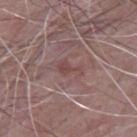Part of a total-body skin-imaging series; this lesion was reviewed on a skin check and was not flagged for biopsy. Longest diameter approximately 3 mm. A male subject, aged around 65. Cropped from a total-body skin-imaging series; the visible field is about 15 mm. On the mid back.